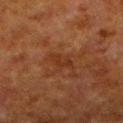notes: imaged on a skin check; not biopsied
image: ~15 mm tile from a whole-body skin photo
subject: male, approximately 80 years of age
diameter: about 3 mm
illumination: cross-polarized illumination
location: the left lower leg
automated metrics: a lesion area of about 3 mm², an eccentricity of roughly 0.9, and a symmetry-axis asymmetry near 0.5; an average lesion color of about L≈25 a*≈20 b*≈27 (CIELAB), about 5 CIELAB-L* units darker than the surrounding skin, and a normalized lesion–skin contrast near 6